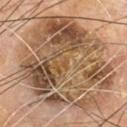Acquisition and patient details: On the chest. A male subject, aged 68–72. The recorded lesion diameter is about 12 mm. Captured under cross-polarized illumination. A close-up tile cropped from a whole-body skin photograph, about 15 mm across.